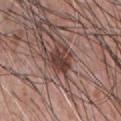A 15 mm close-up tile from a total-body photography series done for melanoma screening.
A male patient in their mid- to late 50s.
On the chest.
Imaged with white-light lighting.